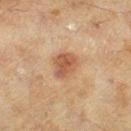Assessment: No biopsy was performed on this lesion — it was imaged during a full skin examination and was not determined to be concerning. Clinical summary: Automated tile analysis of the lesion measured a lesion area of about 7.5 mm², an eccentricity of roughly 0.55, and two-axis asymmetry of about 0.2. The lesion is located on the right lower leg. The subject is a female in their 60s. Longest diameter approximately 3.5 mm. A close-up tile cropped from a whole-body skin photograph, about 15 mm across. Captured under cross-polarized illumination.